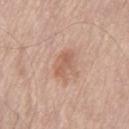<case>
<biopsy_status>not biopsied; imaged during a skin examination</biopsy_status>
<patient>
  <sex>male</sex>
  <age_approx>85+</age_approx>
</patient>
<lighting>white-light</lighting>
<image>
  <source>total-body photography crop</source>
  <field_of_view_mm>15</field_of_view_mm>
</image>
<lesion_size>
  <long_diameter_mm_approx>3.0</long_diameter_mm_approx>
</lesion_size>
<site>leg</site>
</case>